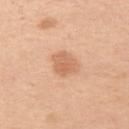<record>
<biopsy_status>not biopsied; imaged during a skin examination</biopsy_status>
<lesion_size>
  <long_diameter_mm_approx>3.0</long_diameter_mm_approx>
</lesion_size>
<patient>
  <sex>female</sex>
  <age_approx>40</age_approx>
</patient>
<site>left upper arm</site>
<image>
  <source>total-body photography crop</source>
  <field_of_view_mm>15</field_of_view_mm>
</image>
<lighting>white-light</lighting>
</record>